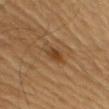Part of a total-body skin-imaging series; this lesion was reviewed on a skin check and was not flagged for biopsy. The lesion is located on the right arm. A male subject, aged 58 to 62. A 15 mm crop from a total-body photograph taken for skin-cancer surveillance.